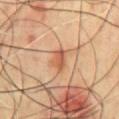workup = total-body-photography surveillance lesion; no biopsy
site = the chest
image-analysis metrics = an average lesion color of about L≈55 a*≈24 b*≈34 (CIELAB), about 10 CIELAB-L* units darker than the surrounding skin, and a normalized lesion–skin contrast near 7; a border-irregularity index near 3/10, a within-lesion color-variation index near 4.5/10, and radial color variation of about 1.5
size = ≈3 mm
tile lighting = cross-polarized illumination
subject = male, roughly 70 years of age
image source = ~15 mm crop, total-body skin-cancer survey